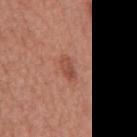Case summary:
* biopsy status — catalogued during a skin exam; not biopsied
* subject — male, approximately 65 years of age
* automated lesion analysis — a footprint of about 4 mm², an eccentricity of roughly 0.85, and a shape-asymmetry score of about 0.25 (0 = symmetric); a color-variation rating of about 3.5/10 and radial color variation of about 1.5; a nevus-likeness score of about 70/100 and a lesion-detection confidence of about 100/100
* image — ~15 mm crop, total-body skin-cancer survey
* body site — the back
* lighting — white-light illumination